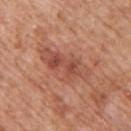Q: Was this lesion biopsied?
A: no biopsy performed (imaged during a skin exam)
Q: What is the imaging modality?
A: total-body-photography crop, ~15 mm field of view
Q: Who is the patient?
A: male, aged 58–62
Q: Illumination type?
A: white-light
Q: Automated lesion metrics?
A: an area of roughly 14 mm², an eccentricity of roughly 0.9, and a symmetry-axis asymmetry near 0.3; a mean CIELAB color near L≈50 a*≈26 b*≈30, roughly 9 lightness units darker than nearby skin, and a lesion-to-skin contrast of about 6.5 (normalized; higher = more distinct); radial color variation of about 1.5
Q: What is the anatomic site?
A: the upper back